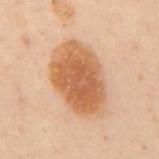Part of a total-body skin-imaging series; this lesion was reviewed on a skin check and was not flagged for biopsy.